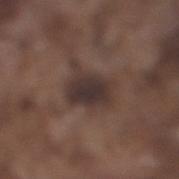Q: Was a biopsy performed?
A: catalogued during a skin exam; not biopsied
Q: How was this image acquired?
A: ~15 mm tile from a whole-body skin photo
Q: How large is the lesion?
A: ≈5.5 mm
Q: Patient demographics?
A: male, in their mid- to late 70s
Q: What is the anatomic site?
A: the leg